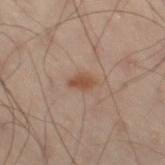Captured during whole-body skin photography for melanoma surveillance; the lesion was not biopsied.
A 15 mm close-up extracted from a 3D total-body photography capture.
Captured under cross-polarized illumination.
The lesion is located on the left leg.
Longest diameter approximately 3 mm.
An algorithmic analysis of the crop reported a color-variation rating of about 1.5/10 and peripheral color asymmetry of about 0.5.
A male patient aged around 50.